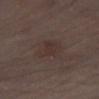Recorded during total-body skin imaging; not selected for excision or biopsy. The patient is a male in their 70s. This is a white-light tile. Approximately 3.5 mm at its widest. An algorithmic analysis of the crop reported an area of roughly 8 mm², an eccentricity of roughly 0.55, and two-axis asymmetry of about 0.25. It also reported internal color variation of about 2.5 on a 0–10 scale and radial color variation of about 1. It also reported an automated nevus-likeness rating near 5 out of 100. A region of skin cropped from a whole-body photographic capture, roughly 15 mm wide. On the left lower leg.